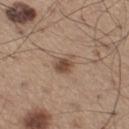Clinical impression:
The lesion was tiled from a total-body skin photograph and was not biopsied.
Image and clinical context:
The patient is a male in their mid- to late 60s. The lesion's longest dimension is about 2.5 mm. Captured under white-light illumination. Automated image analysis of the tile measured a classifier nevus-likeness of about 85/100. The lesion is on the left thigh. A close-up tile cropped from a whole-body skin photograph, about 15 mm across.